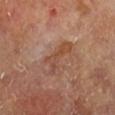Impression:
This lesion was catalogued during total-body skin photography and was not selected for biopsy.
Acquisition and patient details:
The tile uses cross-polarized illumination. The subject is a male approximately 70 years of age. The recorded lesion diameter is about 4.5 mm. On the right lower leg. A close-up tile cropped from a whole-body skin photograph, about 15 mm across.